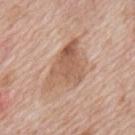Clinical impression:
Captured during whole-body skin photography for melanoma surveillance; the lesion was not biopsied.
Acquisition and patient details:
The patient is a male aged 68 to 72. Captured under white-light illumination. The lesion is on the back. A roughly 15 mm field-of-view crop from a total-body skin photograph. Automated tile analysis of the lesion measured a footprint of about 16 mm², an outline eccentricity of about 0.75 (0 = round, 1 = elongated), and two-axis asymmetry of about 0.5. The software also gave a lesion color around L≈58 a*≈20 b*≈30 in CIELAB, roughly 10 lightness units darker than nearby skin, and a lesion-to-skin contrast of about 7 (normalized; higher = more distinct).